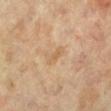biopsy_status: not biopsied; imaged during a skin examination
lesion_size:
  long_diameter_mm_approx: 3.0
image:
  source: total-body photography crop
  field_of_view_mm: 15
lighting: cross-polarized
site: right leg
patient:
  sex: female
  age_approx: 65